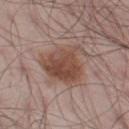Clinical impression:
Part of a total-body skin-imaging series; this lesion was reviewed on a skin check and was not flagged for biopsy.
Image and clinical context:
A male patient aged around 55. On the left thigh. An algorithmic analysis of the crop reported an area of roughly 20 mm² and a symmetry-axis asymmetry near 0.3. The analysis additionally found a lesion color around L≈48 a*≈19 b*≈25 in CIELAB and roughly 11 lightness units darker than nearby skin. It also reported an automated nevus-likeness rating near 85 out of 100 and a lesion-detection confidence of about 100/100. A close-up tile cropped from a whole-body skin photograph, about 15 mm across.